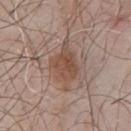The lesion was tiled from a total-body skin photograph and was not biopsied. A lesion tile, about 15 mm wide, cut from a 3D total-body photograph. Captured under white-light illumination. A male subject, aged around 55. The lesion's longest dimension is about 6 mm. On the front of the torso.